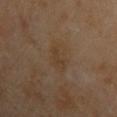No biopsy was performed on this lesion — it was imaged during a full skin examination and was not determined to be concerning.
Cropped from a total-body skin-imaging series; the visible field is about 15 mm.
From the left upper arm.
A male patient aged around 60.